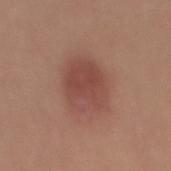<tbp_lesion>
<biopsy_status>not biopsied; imaged during a skin examination</biopsy_status>
<lesion_size>
  <long_diameter_mm_approx>5.0</long_diameter_mm_approx>
</lesion_size>
<patient>
  <sex>male</sex>
  <age_approx>30</age_approx>
</patient>
<lighting>white-light</lighting>
<image>
  <source>total-body photography crop</source>
  <field_of_view_mm>15</field_of_view_mm>
</image>
<site>mid back</site>
</tbp_lesion>